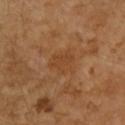The lesion was tiled from a total-body skin photograph and was not biopsied.
This image is a 15 mm lesion crop taken from a total-body photograph.
The patient is a female aged 68 to 72.
From the right forearm.